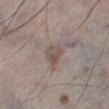Impression:
Part of a total-body skin-imaging series; this lesion was reviewed on a skin check and was not flagged for biopsy.
Context:
A male subject approximately 70 years of age. The lesion is on the left lower leg. The total-body-photography lesion software estimated a footprint of about 4.5 mm², a shape eccentricity near 0.45, and a shape-asymmetry score of about 0.3 (0 = symmetric). The software also gave a mean CIELAB color near L≈51 a*≈12 b*≈21 and a lesion-to-skin contrast of about 6.5 (normalized; higher = more distinct). The analysis additionally found border irregularity of about 2.5 on a 0–10 scale, a color-variation rating of about 4/10, and peripheral color asymmetry of about 1.5. It also reported a nevus-likeness score of about 15/100 and lesion-presence confidence of about 85/100. A roughly 15 mm field-of-view crop from a total-body skin photograph.